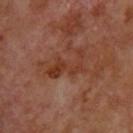The lesion was photographed on a routine skin check and not biopsied; there is no pathology result.
The lesion is located on the upper back.
A male patient, aged around 70.
The recorded lesion diameter is about 5.5 mm.
This is a cross-polarized tile.
This image is a 15 mm lesion crop taken from a total-body photograph.
Automated tile analysis of the lesion measured a mean CIELAB color near L≈36 a*≈24 b*≈30, a lesion–skin lightness drop of about 7, and a lesion-to-skin contrast of about 7 (normalized; higher = more distinct). And it measured a border-irregularity index near 9.5/10, a color-variation rating of about 1.5/10, and radial color variation of about 0.5.